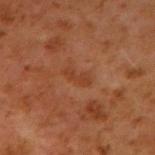Impression: Recorded during total-body skin imaging; not selected for excision or biopsy. Clinical summary: Approximately 3 mm at its widest. A 15 mm close-up tile from a total-body photography series done for melanoma screening. The lesion is located on the right upper arm. A male patient about 60 years old. Automated image analysis of the tile measured a lesion area of about 3 mm², a shape eccentricity near 0.95, and two-axis asymmetry of about 0.35. And it measured a lesion–skin lightness drop of about 6 and a normalized lesion–skin contrast near 5.5. It also reported a nevus-likeness score of about 0/100 and a lesion-detection confidence of about 100/100. This is a cross-polarized tile.